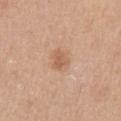The lesion-visualizer software estimated a mean CIELAB color near L≈59 a*≈21 b*≈33, roughly 9 lightness units darker than nearby skin, and a lesion-to-skin contrast of about 6 (normalized; higher = more distinct). And it measured a border-irregularity index near 4/10 and a peripheral color-asymmetry measure near 0.5. The software also gave an automated nevus-likeness rating near 30 out of 100 and lesion-presence confidence of about 100/100. A female subject aged approximately 45. A close-up tile cropped from a whole-body skin photograph, about 15 mm across. On the left upper arm. The tile uses white-light illumination.